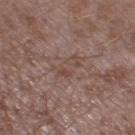Impression: Part of a total-body skin-imaging series; this lesion was reviewed on a skin check and was not flagged for biopsy.